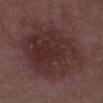Acquisition and patient details:
A 15 mm close-up extracted from a 3D total-body photography capture. The subject is in their 60s. Automated tile analysis of the lesion measured an average lesion color of about L≈31 a*≈20 b*≈17 (CIELAB), a lesion–skin lightness drop of about 8, and a lesion-to-skin contrast of about 7.5 (normalized; higher = more distinct). And it measured a nevus-likeness score of about 35/100 and a lesion-detection confidence of about 100/100. From the front of the torso. Measured at roughly 9.5 mm in maximum diameter. Imaged with white-light lighting.
Conclusion:
Histopathology of the biopsied lesion showed a nodular basal cell carcinoma.